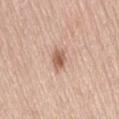The lesion was tiled from a total-body skin photograph and was not biopsied. A lesion tile, about 15 mm wide, cut from a 3D total-body photograph. On the right thigh. A female subject, roughly 65 years of age. An algorithmic analysis of the crop reported a footprint of about 4 mm², an outline eccentricity of about 0.7 (0 = round, 1 = elongated), and a shape-asymmetry score of about 0.2 (0 = symmetric). The analysis additionally found a lesion color around L≈59 a*≈21 b*≈30 in CIELAB and a normalized lesion–skin contrast near 8. The software also gave a within-lesion color-variation index near 3/10 and a peripheral color-asymmetry measure near 1. The software also gave a detector confidence of about 100 out of 100 that the crop contains a lesion. Imaged with white-light lighting. Longest diameter approximately 2.5 mm.